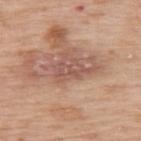biopsy status: no biopsy performed (imaged during a skin exam)
tile lighting: white-light
anatomic site: the upper back
automated lesion analysis: a footprint of about 6 mm², an outline eccentricity of about 0.9 (0 = round, 1 = elongated), and two-axis asymmetry of about 0.35; an average lesion color of about L≈54 a*≈22 b*≈27 (CIELAB), about 9 CIELAB-L* units darker than the surrounding skin, and a normalized border contrast of about 6.5; border irregularity of about 5.5 on a 0–10 scale and peripheral color asymmetry of about 1; a classifier nevus-likeness of about 0/100 and lesion-presence confidence of about 85/100
size: ~4.5 mm (longest diameter)
acquisition: total-body-photography crop, ~15 mm field of view
subject: female, aged around 50